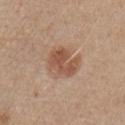Notes:
- follow-up · no biopsy performed (imaged during a skin exam)
- lesion size · ~4 mm (longest diameter)
- TBP lesion metrics · about 10 CIELAB-L* units darker than the surrounding skin and a normalized border contrast of about 7.5; border irregularity of about 3 on a 0–10 scale, a within-lesion color-variation index near 4/10, and radial color variation of about 1.5; a detector confidence of about 100 out of 100 that the crop contains a lesion
- image · ~15 mm crop, total-body skin-cancer survey
- patient · male, approximately 75 years of age
- body site · the right upper arm
- illumination · white-light